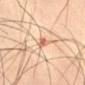Assessment: No biopsy was performed on this lesion — it was imaged during a full skin examination and was not determined to be concerning. Acquisition and patient details: The recorded lesion diameter is about 3 mm. A close-up tile cropped from a whole-body skin photograph, about 15 mm across. Located on the lower back. A male subject, roughly 40 years of age. Captured under cross-polarized illumination.